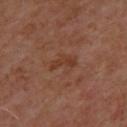acquisition: 15 mm crop, total-body photography | patient: male, in their 50s | image-analysis metrics: a lesion area of about 3 mm² and two-axis asymmetry of about 0.4; about 6 CIELAB-L* units darker than the surrounding skin and a normalized border contrast of about 6; a border-irregularity rating of about 4.5/10 and a within-lesion color-variation index near 0/10; a nevus-likeness score of about 0/100 and a detector confidence of about 100 out of 100 that the crop contains a lesion | tile lighting: cross-polarized illumination | size: ≈3 mm | site: the upper back.